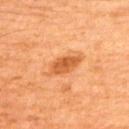Recorded during total-body skin imaging; not selected for excision or biopsy. This image is a 15 mm lesion crop taken from a total-body photograph. On the back. The subject is a male roughly 65 years of age.